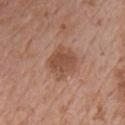biopsy status — catalogued during a skin exam; not biopsied | subject — male, aged 73–77 | acquisition — 15 mm crop, total-body photography | illumination — white-light | anatomic site — the chest | lesion size — about 3.5 mm | automated metrics — a lesion-detection confidence of about 100/100.